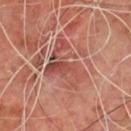notes = imaged on a skin check; not biopsied
TBP lesion metrics = an area of roughly 16 mm² and a symmetry-axis asymmetry near 0.55; border irregularity of about 9 on a 0–10 scale and peripheral color asymmetry of about 2.5; an automated nevus-likeness rating near 0 out of 100 and a lesion-detection confidence of about 80/100
acquisition = ~15 mm crop, total-body skin-cancer survey
subject = male, aged approximately 65
lighting = cross-polarized illumination
anatomic site = the chest
lesion diameter = ≈6.5 mm